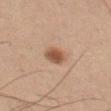notes: catalogued during a skin exam; not biopsied
patient: male, approximately 65 years of age
location: the left thigh
illumination: cross-polarized
size: ~2.5 mm (longest diameter)
acquisition: ~15 mm tile from a whole-body skin photo